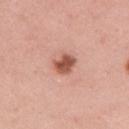This lesion was catalogued during total-body skin photography and was not selected for biopsy. Automated tile analysis of the lesion measured a lesion area of about 5 mm², a shape eccentricity near 0.6, and a shape-asymmetry score of about 0.25 (0 = symmetric). And it measured a nevus-likeness score of about 95/100 and a lesion-detection confidence of about 100/100. A female patient, in their mid-30s. Measured at roughly 2.5 mm in maximum diameter. From the right upper arm. Imaged with white-light lighting. A 15 mm close-up extracted from a 3D total-body photography capture.